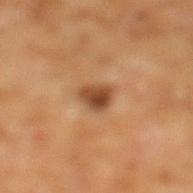No biopsy was performed on this lesion — it was imaged during a full skin examination and was not determined to be concerning. Captured under cross-polarized illumination. The lesion's longest dimension is about 2.5 mm. A 15 mm close-up tile from a total-body photography series done for melanoma screening. The subject is a male aged around 60. The lesion is located on the left lower leg.